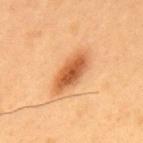follow-up: imaged on a skin check; not biopsied | body site: the upper back | image source: ~15 mm crop, total-body skin-cancer survey | size: ≈6.5 mm | subject: male, aged 53–57.